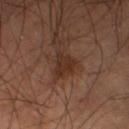Notes:
- biopsy status · catalogued during a skin exam; not biopsied
- illumination · cross-polarized
- anatomic site · the left thigh
- subject · male, aged around 55
- acquisition · ~15 mm crop, total-body skin-cancer survey
- automated lesion analysis · a mean CIELAB color near L≈27 a*≈17 b*≈23, a lesion–skin lightness drop of about 7, and a normalized border contrast of about 7.5; a border-irregularity rating of about 4/10 and peripheral color asymmetry of about 0.5